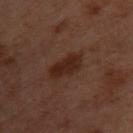Notes:
* workup · total-body-photography surveillance lesion; no biopsy
* anatomic site · the back
* subject · female, approximately 55 years of age
* automated lesion analysis · an area of roughly 8 mm², a shape eccentricity near 0.9, and two-axis asymmetry of about 0.2; a mean CIELAB color near L≈20 a*≈16 b*≈20, roughly 7 lightness units darker than nearby skin, and a lesion-to-skin contrast of about 8.5 (normalized; higher = more distinct); a border-irregularity index near 2/10, a within-lesion color-variation index near 2/10, and radial color variation of about 0.5; an automated nevus-likeness rating near 55 out of 100 and a detector confidence of about 100 out of 100 that the crop contains a lesion
* acquisition · ~15 mm crop, total-body skin-cancer survey
* illumination · cross-polarized illumination